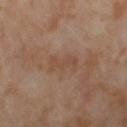Imaged during a routine full-body skin examination; the lesion was not biopsied and no histopathology is available. Automated tile analysis of the lesion measured a shape eccentricity near 0.85 and a symmetry-axis asymmetry near 0.4. The analysis additionally found a color-variation rating of about 1/10 and a peripheral color-asymmetry measure near 0.5. The analysis additionally found an automated nevus-likeness rating near 0 out of 100. Measured at roughly 3 mm in maximum diameter. A 15 mm crop from a total-body photograph taken for skin-cancer surveillance. A female subject aged 53–57. The tile uses cross-polarized illumination. On the right thigh.